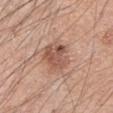lesion size — ~4 mm (longest diameter) | image source — 15 mm crop, total-body photography | subject — male, roughly 45 years of age | tile lighting — white-light illumination | body site — the arm.